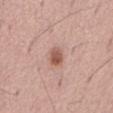This lesion was catalogued during total-body skin photography and was not selected for biopsy. The lesion is on the abdomen. A region of skin cropped from a whole-body photographic capture, roughly 15 mm wide. An algorithmic analysis of the crop reported a border-irregularity index near 1.5/10, internal color variation of about 4 on a 0–10 scale, and a peripheral color-asymmetry measure near 1.5. About 2.5 mm across. The subject is a male about 75 years old. This is a white-light tile.